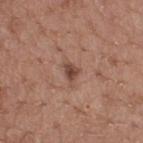Part of a total-body skin-imaging series; this lesion was reviewed on a skin check and was not flagged for biopsy. Measured at roughly 2.5 mm in maximum diameter. The lesion-visualizer software estimated a footprint of about 3.5 mm² and an eccentricity of roughly 0.65. It also reported a border-irregularity rating of about 3.5/10 and peripheral color asymmetry of about 1. The tile uses white-light illumination. A close-up tile cropped from a whole-body skin photograph, about 15 mm across. The lesion is located on the upper back. The subject is a male aged approximately 65.